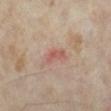notes — total-body-photography surveillance lesion; no biopsy
patient — female, in their mid- to late 30s
site — the leg
image-analysis metrics — a lesion color around L≈52 a*≈24 b*≈25 in CIELAB, a lesion–skin lightness drop of about 8, and a normalized border contrast of about 6; an automated nevus-likeness rating near 0 out of 100
size — ≈2.5 mm
acquisition — 15 mm crop, total-body photography
tile lighting — cross-polarized illumination